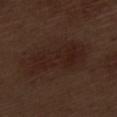No biopsy was performed on this lesion — it was imaged during a full skin examination and was not determined to be concerning.